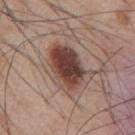biopsy status: total-body-photography surveillance lesion; no biopsy
automated metrics: a lesion area of about 16 mm² and an outline eccentricity of about 0.8 (0 = round, 1 = elongated); a mean CIELAB color near L≈42 a*≈20 b*≈23; a lesion-detection confidence of about 100/100
site: the mid back
lesion size: about 6 mm
acquisition: ~15 mm tile from a whole-body skin photo
patient: male, roughly 55 years of age